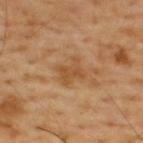This lesion was catalogued during total-body skin photography and was not selected for biopsy.
On the upper back.
The tile uses cross-polarized illumination.
The lesion's longest dimension is about 3 mm.
Automated image analysis of the tile measured an area of roughly 6.5 mm², an outline eccentricity of about 0.6 (0 = round, 1 = elongated), and a symmetry-axis asymmetry near 0.25. The software also gave a color-variation rating of about 2.5/10 and a peripheral color-asymmetry measure near 1. The software also gave a detector confidence of about 100 out of 100 that the crop contains a lesion.
A region of skin cropped from a whole-body photographic capture, roughly 15 mm wide.
The patient is a male aged around 55.